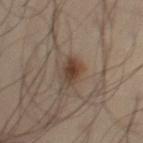acquisition: ~15 mm tile from a whole-body skin photo | illumination: cross-polarized | image-analysis metrics: an outline eccentricity of about 0.4 (0 = round, 1 = elongated) and a symmetry-axis asymmetry near 0.2; a border-irregularity rating of about 2/10, internal color variation of about 5.5 on a 0–10 scale, and peripheral color asymmetry of about 2; a nevus-likeness score of about 95/100 and a lesion-detection confidence of about 100/100 | patient: male, aged 48–52 | location: the lower back.